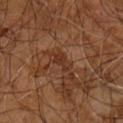Q: Automated lesion metrics?
A: an area of roughly 2.5 mm² and a shape-asymmetry score of about 0.45 (0 = symmetric)
Q: How large is the lesion?
A: ~2.5 mm (longest diameter)
Q: How was the tile lit?
A: cross-polarized illumination
Q: What are the patient's age and sex?
A: male, about 60 years old
Q: How was this image acquired?
A: total-body-photography crop, ~15 mm field of view
Q: What is the anatomic site?
A: the right arm